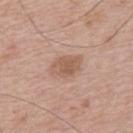Q: Is there a histopathology result?
A: imaged on a skin check; not biopsied
Q: How was the tile lit?
A: white-light
Q: What is the imaging modality?
A: 15 mm crop, total-body photography
Q: What is the anatomic site?
A: the mid back
Q: Patient demographics?
A: male, aged around 65
Q: Lesion size?
A: ~4 mm (longest diameter)
Q: Automated lesion metrics?
A: a footprint of about 7.5 mm², an outline eccentricity of about 0.7 (0 = round, 1 = elongated), and a symmetry-axis asymmetry near 0.25; lesion-presence confidence of about 100/100